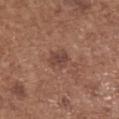{
  "biopsy_status": "not biopsied; imaged during a skin examination",
  "site": "upper back",
  "image": {
    "source": "total-body photography crop",
    "field_of_view_mm": 15
  },
  "patient": {
    "sex": "female",
    "age_approx": 75
  },
  "lighting": "white-light"
}